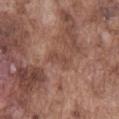TBP lesion metrics: an area of roughly 4 mm², a shape eccentricity near 0.85, and a shape-asymmetry score of about 0.3 (0 = symmetric); a lesion color around L≈46 a*≈21 b*≈26 in CIELAB and a normalized lesion–skin contrast near 5; a color-variation rating of about 2.5/10 and radial color variation of about 1
image source: ~15 mm crop, total-body skin-cancer survey
tile lighting: white-light
diameter: ≈3 mm
patient: male, aged 73–77
body site: the abdomen